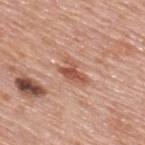Case summary:
* image source — ~15 mm crop, total-body skin-cancer survey
* automated metrics — an area of roughly 5 mm², an outline eccentricity of about 0.85 (0 = round, 1 = elongated), and a symmetry-axis asymmetry near 0.5; an average lesion color of about L≈54 a*≈25 b*≈31 (CIELAB), a lesion–skin lightness drop of about 11, and a normalized border contrast of about 8; border irregularity of about 5.5 on a 0–10 scale, a color-variation rating of about 2/10, and peripheral color asymmetry of about 0.5
* lighting — white-light
* anatomic site — the upper back
* patient — male, aged 78 to 82
* diameter — ~3.5 mm (longest diameter)